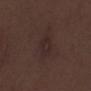workup: catalogued during a skin exam; not biopsied | lighting: white-light illumination | image source: ~15 mm crop, total-body skin-cancer survey | patient: male, approximately 70 years of age | diameter: about 3 mm | anatomic site: the left lower leg.